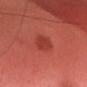Q: Was this lesion biopsied?
A: no biopsy performed (imaged during a skin exam)
Q: How was this image acquired?
A: ~15 mm crop, total-body skin-cancer survey
Q: What are the patient's age and sex?
A: female, in their mid- to late 40s
Q: Where on the body is the lesion?
A: the head or neck